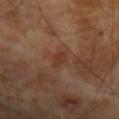Case summary:
• notes: imaged on a skin check; not biopsied
• illumination: cross-polarized illumination
• location: the left forearm
• acquisition: ~15 mm crop, total-body skin-cancer survey
• patient: male, about 70 years old
• size: ≈2.5 mm